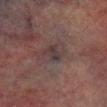Assessment:
The lesion was photographed on a routine skin check and not biopsied; there is no pathology result.
Background:
A male patient, approximately 75 years of age. This image is a 15 mm lesion crop taken from a total-body photograph. The lesion is located on the right lower leg.